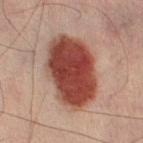Case summary:
– lighting · cross-polarized
– body site · the left lower leg
– lesion diameter · about 8.5 mm
– image source · ~15 mm crop, total-body skin-cancer survey
– patient · male, in their 50s